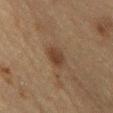Imaged during a routine full-body skin examination; the lesion was not biopsied and no histopathology is available. Longest diameter approximately 4 mm. Located on the chest. The patient is a male in their mid-70s. A 15 mm close-up tile from a total-body photography series done for melanoma screening.